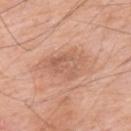This lesion was catalogued during total-body skin photography and was not selected for biopsy.